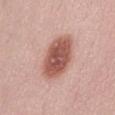Case summary:
– notes · no biopsy performed (imaged during a skin exam)
– diameter · about 6.5 mm
– subject · female, about 25 years old
– tile lighting · white-light illumination
– acquisition · total-body-photography crop, ~15 mm field of view
– TBP lesion metrics · a shape-asymmetry score of about 0.15 (0 = symmetric); an average lesion color of about L≈55 a*≈25 b*≈26 (CIELAB), about 16 CIELAB-L* units darker than the surrounding skin, and a lesion-to-skin contrast of about 10 (normalized; higher = more distinct); border irregularity of about 2 on a 0–10 scale, a color-variation rating of about 4/10, and peripheral color asymmetry of about 1; an automated nevus-likeness rating near 95 out of 100 and lesion-presence confidence of about 100/100
– location · the abdomen